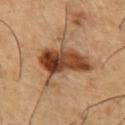  biopsy_status: not biopsied; imaged during a skin examination
  site: chest
  patient:
    sex: male
    age_approx: 55
  image:
    source: total-body photography crop
    field_of_view_mm: 15
  lighting: cross-polarized